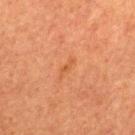Impression:
Imaged during a routine full-body skin examination; the lesion was not biopsied and no histopathology is available.
Image and clinical context:
About 2.5 mm across. A male subject in their 50s. An algorithmic analysis of the crop reported a shape eccentricity near 0.95 and a symmetry-axis asymmetry near 0.5. It also reported a lesion color around L≈45 a*≈24 b*≈35 in CIELAB and a normalized lesion–skin contrast near 5. The analysis additionally found an automated nevus-likeness rating near 0 out of 100 and lesion-presence confidence of about 100/100. Captured under cross-polarized illumination. On the mid back. A 15 mm close-up extracted from a 3D total-body photography capture.